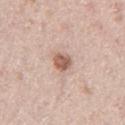workup = total-body-photography surveillance lesion; no biopsy | site = the left thigh | subject = male, in their mid-60s | size = about 2.5 mm | image source = ~15 mm tile from a whole-body skin photo.